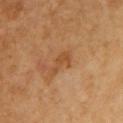<case>
<biopsy_status>not biopsied; imaged during a skin examination</biopsy_status>
<image>
  <source>total-body photography crop</source>
  <field_of_view_mm>15</field_of_view_mm>
</image>
<lighting>cross-polarized</lighting>
<site>chest</site>
<patient>
  <sex>female</sex>
  <age_approx>55</age_approx>
</patient>
<lesion_size>
  <long_diameter_mm_approx>3.0</long_diameter_mm_approx>
</lesion_size>
</case>